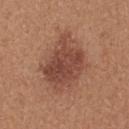<tbp_lesion>
<biopsy_status>not biopsied; imaged during a skin examination</biopsy_status>
<automated_metrics>
  <eccentricity>0.65</eccentricity>
  <shape_asymmetry>0.3</shape_asymmetry>
  <vs_skin_darker_L>11.0</vs_skin_darker_L>
  <vs_skin_contrast_norm>8.5</vs_skin_contrast_norm>
  <border_irregularity_0_10>3.5</border_irregularity_0_10>
  <color_variation_0_10>4.0</color_variation_0_10>
  <peripheral_color_asymmetry>1.5</peripheral_color_asymmetry>
</automated_metrics>
<site>back</site>
<patient>
  <sex>female</sex>
  <age_approx>40</age_approx>
</patient>
<image>
  <source>total-body photography crop</source>
  <field_of_view_mm>15</field_of_view_mm>
</image>
</tbp_lesion>